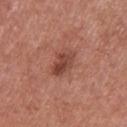Impression: Imaged during a routine full-body skin examination; the lesion was not biopsied and no histopathology is available. Clinical summary: The lesion is on the upper back. The patient is a female aged approximately 40. Captured under white-light illumination. Longest diameter approximately 3.5 mm. An algorithmic analysis of the crop reported a lesion area of about 5.5 mm², an eccentricity of roughly 0.8, and two-axis asymmetry of about 0.25. It also reported an average lesion color of about L≈44 a*≈25 b*≈27 (CIELAB) and a normalized lesion–skin contrast near 8. The analysis additionally found a border-irregularity rating of about 2.5/10, internal color variation of about 4.5 on a 0–10 scale, and a peripheral color-asymmetry measure near 1.5. Cropped from a whole-body photographic skin survey; the tile spans about 15 mm.